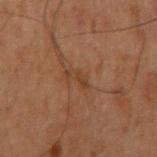Captured during whole-body skin photography for melanoma surveillance; the lesion was not biopsied.
The patient is a male aged approximately 60.
The recorded lesion diameter is about 3 mm.
Automated image analysis of the tile measured a footprint of about 4 mm², a shape eccentricity near 0.85, and two-axis asymmetry of about 0.55. It also reported a nevus-likeness score of about 0/100 and a lesion-detection confidence of about 90/100.
The lesion is located on the right upper arm.
This image is a 15 mm lesion crop taken from a total-body photograph.